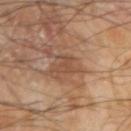<tbp_lesion>
  <lighting>cross-polarized</lighting>
  <lesion_size>
    <long_diameter_mm_approx>3.0</long_diameter_mm_approx>
  </lesion_size>
  <site>right upper arm</site>
  <automated_metrics>
    <area_mm2_approx>4.0</area_mm2_approx>
    <eccentricity>0.75</eccentricity>
    <shape_asymmetry>0.55</shape_asymmetry>
    <border_irregularity_0_10>6.0</border_irregularity_0_10>
    <color_variation_0_10>1.0</color_variation_0_10>
    <peripheral_color_asymmetry>0.5</peripheral_color_asymmetry>
  </automated_metrics>
  <image>
    <source>total-body photography crop</source>
    <field_of_view_mm>15</field_of_view_mm>
  </image>
  <patient>
    <sex>male</sex>
    <age_approx>65</age_approx>
  </patient>
</tbp_lesion>